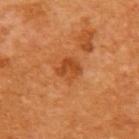  biopsy_status: not biopsied; imaged during a skin examination
  image:
    source: total-body photography crop
    field_of_view_mm: 15
  patient:
    sex: female
    age_approx: 55
  site: upper back